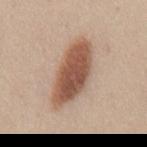| key | value |
|---|---|
| biopsy status | no biopsy performed (imaged during a skin exam) |
| patient | female, in their 40s |
| anatomic site | the mid back |
| TBP lesion metrics | a lesion color around L≈53 a*≈20 b*≈29 in CIELAB and roughly 16 lightness units darker than nearby skin; a nevus-likeness score of about 100/100 and a lesion-detection confidence of about 100/100 |
| lesion diameter | about 8 mm |
| imaging modality | total-body-photography crop, ~15 mm field of view |
| illumination | white-light illumination |